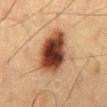workup: total-body-photography surveillance lesion; no biopsy
subject: male, aged approximately 60
illumination: cross-polarized illumination
acquisition: ~15 mm tile from a whole-body skin photo
anatomic site: the back
size: ~6 mm (longest diameter)